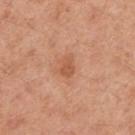No biopsy was performed on this lesion — it was imaged during a full skin examination and was not determined to be concerning. The lesion is on the arm. An algorithmic analysis of the crop reported an area of roughly 4 mm² and a shape-asymmetry score of about 0.25 (0 = symmetric). And it measured a lesion color around L≈56 a*≈25 b*≈35 in CIELAB, about 8 CIELAB-L* units darker than the surrounding skin, and a normalized lesion–skin contrast near 6. Cropped from a whole-body photographic skin survey; the tile spans about 15 mm. The subject is a female aged 38–42. The tile uses white-light illumination. The recorded lesion diameter is about 2.5 mm.